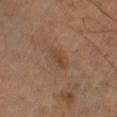| feature | finding |
|---|---|
| notes | no biopsy performed (imaged during a skin exam) |
| diameter | about 3 mm |
| body site | the right leg |
| tile lighting | cross-polarized |
| patient | male, aged around 60 |
| acquisition | 15 mm crop, total-body photography |